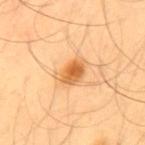Assessment: Part of a total-body skin-imaging series; this lesion was reviewed on a skin check and was not flagged for biopsy. Context: A lesion tile, about 15 mm wide, cut from a 3D total-body photograph. The patient is a male approximately 55 years of age. This is a cross-polarized tile. Automated image analysis of the tile measured a border-irregularity index near 2.5/10, a color-variation rating of about 5/10, and a peripheral color-asymmetry measure near 1.5. The lesion is located on the mid back. The lesion's longest dimension is about 3.5 mm.